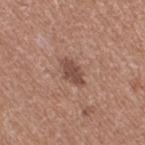Clinical impression: Captured during whole-body skin photography for melanoma surveillance; the lesion was not biopsied. Acquisition and patient details: This image is a 15 mm lesion crop taken from a total-body photograph. Located on the leg. A female subject roughly 35 years of age. The recorded lesion diameter is about 3.5 mm.